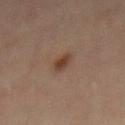Q: Was a biopsy performed?
A: total-body-photography surveillance lesion; no biopsy
Q: Lesion location?
A: the mid back
Q: What is the imaging modality?
A: ~15 mm tile from a whole-body skin photo
Q: Patient demographics?
A: male, aged 43–47